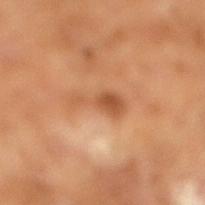No biopsy was performed on this lesion — it was imaged during a full skin examination and was not determined to be concerning.
A male subject aged approximately 65.
Cropped from a total-body skin-imaging series; the visible field is about 15 mm.